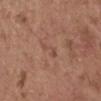Acquisition and patient details:
The lesion is on the arm. A female patient, approximately 65 years of age. Captured under white-light illumination. Approximately 2.5 mm at its widest. A close-up tile cropped from a whole-body skin photograph, about 15 mm across.